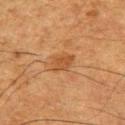lesion_size:
  long_diameter_mm_approx: 2.5
site: left upper arm
patient:
  sex: male
  age_approx: 50
image:
  source: total-body photography crop
  field_of_view_mm: 15
lighting: cross-polarized